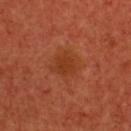Q: Was this lesion biopsied?
A: catalogued during a skin exam; not biopsied
Q: How was the tile lit?
A: cross-polarized illumination
Q: Automated lesion metrics?
A: a lesion area of about 8.5 mm², an eccentricity of roughly 0.4, and a symmetry-axis asymmetry near 0.25; an average lesion color of about L≈39 a*≈29 b*≈37 (CIELAB), a lesion–skin lightness drop of about 6, and a normalized lesion–skin contrast near 6; a border-irregularity index near 2.5/10, a color-variation rating of about 2/10, and radial color variation of about 0.5
Q: How was this image acquired?
A: 15 mm crop, total-body photography
Q: Lesion size?
A: ~3.5 mm (longest diameter)
Q: What are the patient's age and sex?
A: female, aged 48–52